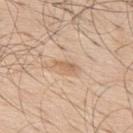Q: Was a biopsy performed?
A: total-body-photography surveillance lesion; no biopsy
Q: How was the tile lit?
A: white-light
Q: Lesion size?
A: ~2.5 mm (longest diameter)
Q: What is the anatomic site?
A: the upper back
Q: Patient demographics?
A: male, approximately 70 years of age
Q: How was this image acquired?
A: total-body-photography crop, ~15 mm field of view